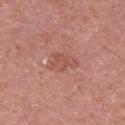Captured during whole-body skin photography for melanoma surveillance; the lesion was not biopsied.
A female patient, in their 50s.
On the right upper arm.
A 15 mm close-up tile from a total-body photography series done for melanoma screening.